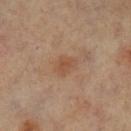follow-up=total-body-photography surveillance lesion; no biopsy
location=the leg
subject=female, aged around 65
imaging modality=~15 mm crop, total-body skin-cancer survey